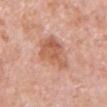* workup · imaged on a skin check; not biopsied
* acquisition · 15 mm crop, total-body photography
* body site · the left upper arm
* lesion size · ≈6 mm
* illumination · white-light
* patient · male, roughly 80 years of age
* automated metrics · an average lesion color of about L≈62 a*≈23 b*≈31 (CIELAB), about 8 CIELAB-L* units darker than the surrounding skin, and a lesion-to-skin contrast of about 6 (normalized; higher = more distinct); a border-irregularity rating of about 3/10 and internal color variation of about 5.5 on a 0–10 scale